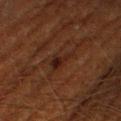Recorded during total-body skin imaging; not selected for excision or biopsy. A male subject, in their 60s. The lesion is located on the right lower leg. About 4 mm across. The tile uses cross-polarized illumination. A 15 mm close-up tile from a total-body photography series done for melanoma screening.